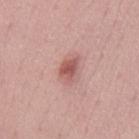The lesion was tiled from a total-body skin photograph and was not biopsied. The lesion is on the back. Captured under white-light illumination. A region of skin cropped from a whole-body photographic capture, roughly 15 mm wide. The lesion's longest dimension is about 3 mm. The subject is a male about 50 years old.